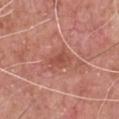Part of a total-body skin-imaging series; this lesion was reviewed on a skin check and was not flagged for biopsy.
A male patient, in their 60s.
Longest diameter approximately 3 mm.
A region of skin cropped from a whole-body photographic capture, roughly 15 mm wide.
On the chest.
This is a white-light tile.
Automated image analysis of the tile measured a classifier nevus-likeness of about 0/100 and a lesion-detection confidence of about 100/100.